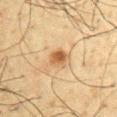Part of a total-body skin-imaging series; this lesion was reviewed on a skin check and was not flagged for biopsy. The lesion is located on the chest. A male subject, approximately 60 years of age. A roughly 15 mm field-of-view crop from a total-body skin photograph.